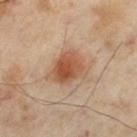This lesion was catalogued during total-body skin photography and was not selected for biopsy. The recorded lesion diameter is about 4.5 mm. A male patient, about 55 years old. Cropped from a whole-body photographic skin survey; the tile spans about 15 mm. Captured under cross-polarized illumination. Automated image analysis of the tile measured an eccentricity of roughly 0.55 and a shape-asymmetry score of about 0.25 (0 = symmetric). It also reported a lesion color around L≈54 a*≈22 b*≈33 in CIELAB and a lesion–skin lightness drop of about 12. The analysis additionally found a border-irregularity rating of about 2.5/10 and internal color variation of about 5.5 on a 0–10 scale. And it measured a lesion-detection confidence of about 100/100. On the left thigh.